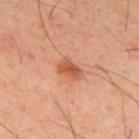Assessment:
This lesion was catalogued during total-body skin photography and was not selected for biopsy.
Acquisition and patient details:
Imaged with cross-polarized lighting. The patient is a male about 40 years old. Located on the mid back. This image is a 15 mm lesion crop taken from a total-body photograph.